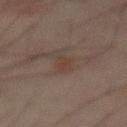The lesion was tiled from a total-body skin photograph and was not biopsied. A male patient approximately 70 years of age. Cropped from a total-body skin-imaging series; the visible field is about 15 mm. The lesion is located on the mid back. The total-body-photography lesion software estimated a mean CIELAB color near L≈31 a*≈13 b*≈19, a lesion–skin lightness drop of about 4, and a lesion-to-skin contrast of about 5 (normalized; higher = more distinct). The software also gave a border-irregularity rating of about 5/10, a color-variation rating of about 1/10, and peripheral color asymmetry of about 0.